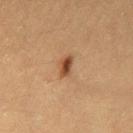– biopsy status — catalogued during a skin exam; not biopsied
– anatomic site — the left thigh
– illumination — cross-polarized
– TBP lesion metrics — a normalized border contrast of about 10
– subject — female, aged approximately 30
– image — ~15 mm tile from a whole-body skin photo